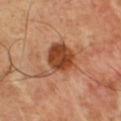Imaged with cross-polarized lighting. The lesion is located on the chest. A 15 mm crop from a total-body photograph taken for skin-cancer surveillance. A male subject roughly 50 years of age. Longest diameter approximately 6 mm.